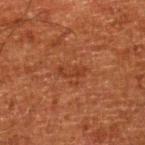<case>
<biopsy_status>not biopsied; imaged during a skin examination</biopsy_status>
<patient>
  <sex>male</sex>
  <age_approx>80</age_approx>
</patient>
<image>
  <source>total-body photography crop</source>
  <field_of_view_mm>15</field_of_view_mm>
</image>
<site>right lower leg</site>
</case>